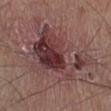biopsy status = catalogued during a skin exam; not biopsied | subject = male, in their mid- to late 50s | imaging modality = ~15 mm tile from a whole-body skin photo | location = the right lower leg | tile lighting = white-light illumination.